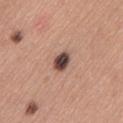Clinical impression:
The lesion was tiled from a total-body skin photograph and was not biopsied.
Clinical summary:
Imaged with white-light lighting. A female subject, aged around 30. The lesion-visualizer software estimated a lesion area of about 4.5 mm² and a shape eccentricity near 0.8. The software also gave a mean CIELAB color near L≈45 a*≈18 b*≈22 and about 20 CIELAB-L* units darker than the surrounding skin. The software also gave a border-irregularity rating of about 2/10 and radial color variation of about 2. The analysis additionally found an automated nevus-likeness rating near 55 out of 100 and lesion-presence confidence of about 100/100. Located on the left thigh. A 15 mm crop from a total-body photograph taken for skin-cancer surveillance. Approximately 3 mm at its widest.